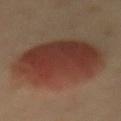biopsy_status: not biopsied; imaged during a skin examination
image:
  source: total-body photography crop
  field_of_view_mm: 15
site: mid back
automated_metrics:
  cielab_L: 37
  cielab_a: 23
  cielab_b: 26
  vs_skin_darker_L: 11.0
  border_irregularity_0_10: 2.0
lighting: cross-polarized
patient:
  sex: female
  age_approx: 60
lesion_size:
  long_diameter_mm_approx: 9.5